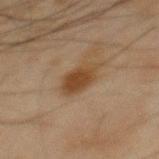No biopsy was performed on this lesion — it was imaged during a full skin examination and was not determined to be concerning.
A lesion tile, about 15 mm wide, cut from a 3D total-body photograph.
From the mid back.
Measured at roughly 4 mm in maximum diameter.
Captured under cross-polarized illumination.
A male patient, in their mid-40s.